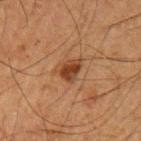| feature | finding |
|---|---|
| notes | catalogued during a skin exam; not biopsied |
| size | about 3 mm |
| body site | the arm |
| patient | male, approximately 65 years of age |
| imaging modality | ~15 mm crop, total-body skin-cancer survey |
| illumination | cross-polarized illumination |